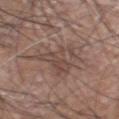No biopsy was performed on this lesion — it was imaged during a full skin examination and was not determined to be concerning.
Longest diameter approximately 9 mm.
On the head or neck.
A male subject aged around 60.
This is a white-light tile.
Cropped from a whole-body photographic skin survey; the tile spans about 15 mm.